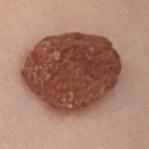This lesion was catalogued during total-body skin photography and was not selected for biopsy.
Longest diameter approximately 11 mm.
The tile uses cross-polarized illumination.
From the left arm.
A male patient aged 48–52.
A region of skin cropped from a whole-body photographic capture, roughly 15 mm wide.